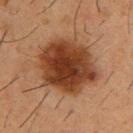Q: Was this lesion biopsied?
A: no biopsy performed (imaged during a skin exam)
Q: What is the imaging modality?
A: ~15 mm tile from a whole-body skin photo
Q: Automated lesion metrics?
A: an eccentricity of roughly 0.5 and two-axis asymmetry of about 0.1; a mean CIELAB color near L≈34 a*≈21 b*≈30, a lesion–skin lightness drop of about 14, and a lesion-to-skin contrast of about 12.5 (normalized; higher = more distinct)
Q: Lesion location?
A: the chest
Q: Who is the patient?
A: male, in their mid- to late 50s
Q: What lighting was used for the tile?
A: cross-polarized
Q: How large is the lesion?
A: about 7.5 mm